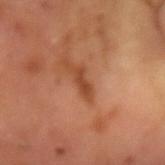A close-up tile cropped from a whole-body skin photograph, about 15 mm across.
A male subject, aged 63–67.